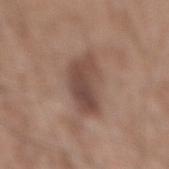Q: Is there a histopathology result?
A: catalogued during a skin exam; not biopsied
Q: Who is the patient?
A: male, approximately 60 years of age
Q: What is the imaging modality?
A: ~15 mm tile from a whole-body skin photo
Q: Lesion location?
A: the mid back